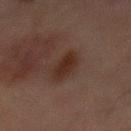The lesion was photographed on a routine skin check and not biopsied; there is no pathology result.
Located on the abdomen.
The subject is a male aged around 65.
A 15 mm close-up tile from a total-body photography series done for melanoma screening.